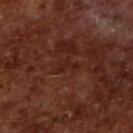Clinical impression: Part of a total-body skin-imaging series; this lesion was reviewed on a skin check and was not flagged for biopsy. Background: A lesion tile, about 15 mm wide, cut from a 3D total-body photograph. A male patient, aged 63 to 67. The tile uses cross-polarized illumination. Automated image analysis of the tile measured a border-irregularity rating of about 7/10 and internal color variation of about 0 on a 0–10 scale.